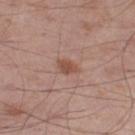Impression:
No biopsy was performed on this lesion — it was imaged during a full skin examination and was not determined to be concerning.